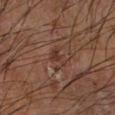biopsy status = catalogued during a skin exam; not biopsied | tile lighting = cross-polarized illumination | image source = 15 mm crop, total-body photography | automated metrics = an eccentricity of roughly 0.7 and two-axis asymmetry of about 0.55; border irregularity of about 6 on a 0–10 scale and peripheral color asymmetry of about 0.5 | site = the left forearm | diameter = ~2.5 mm (longest diameter) | patient = male, aged 63 to 67.